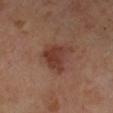lesion diameter=≈4.5 mm | site=the leg | image=15 mm crop, total-body photography | subject=male, aged 68–72.